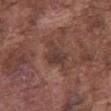biopsy status = catalogued during a skin exam; not biopsied